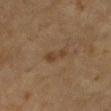Q: Was this lesion biopsied?
A: no biopsy performed (imaged during a skin exam)
Q: What is the anatomic site?
A: the left forearm
Q: What are the patient's age and sex?
A: female, roughly 80 years of age
Q: How large is the lesion?
A: ≈2.5 mm
Q: How was this image acquired?
A: total-body-photography crop, ~15 mm field of view